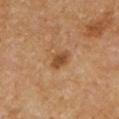Recorded during total-body skin imaging; not selected for excision or biopsy.
On the arm.
About 3 mm across.
A lesion tile, about 15 mm wide, cut from a 3D total-body photograph.
A male patient in their mid- to late 50s.
An algorithmic analysis of the crop reported a shape eccentricity near 0.65. The analysis additionally found border irregularity of about 2 on a 0–10 scale.